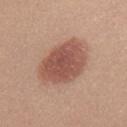Case summary:
- diameter: about 7 mm
- image source: total-body-photography crop, ~15 mm field of view
- site: the upper back
- tile lighting: white-light illumination
- patient: female, aged 33–37